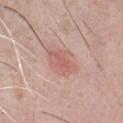Impression: Imaged during a routine full-body skin examination; the lesion was not biopsied and no histopathology is available. Clinical summary: Captured under white-light illumination. A male patient aged 48–52. An algorithmic analysis of the crop reported a lesion area of about 7.5 mm², a shape eccentricity near 0.7, and a shape-asymmetry score of about 0.25 (0 = symmetric). The software also gave a lesion–skin lightness drop of about 8 and a lesion-to-skin contrast of about 5.5 (normalized; higher = more distinct). The analysis additionally found an automated nevus-likeness rating near 85 out of 100 and lesion-presence confidence of about 100/100. Longest diameter approximately 4 mm. The lesion is on the chest. A roughly 15 mm field-of-view crop from a total-body skin photograph.